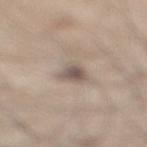Impression:
The lesion was tiled from a total-body skin photograph and was not biopsied.
Context:
A roughly 15 mm field-of-view crop from a total-body skin photograph. The subject is a male about 45 years old. The tile uses white-light illumination. Automated tile analysis of the lesion measured a footprint of about 5 mm², a shape eccentricity near 0.65, and two-axis asymmetry of about 0.3. The analysis additionally found a border-irregularity index near 3/10 and peripheral color asymmetry of about 1. The software also gave a nevus-likeness score of about 10/100 and a detector confidence of about 75 out of 100 that the crop contains a lesion. From the right lower leg.